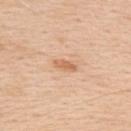Findings:
- workup · imaged on a skin check; not biopsied